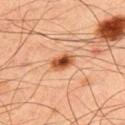Clinical impression: The lesion was photographed on a routine skin check and not biopsied; there is no pathology result. Context: The lesion is located on the upper back. The lesion-visualizer software estimated roughly 17 lightness units darker than nearby skin and a lesion-to-skin contrast of about 11 (normalized; higher = more distinct). The analysis additionally found border irregularity of about 1.5 on a 0–10 scale and a color-variation rating of about 5.5/10. The software also gave lesion-presence confidence of about 100/100. Imaged with cross-polarized lighting. A male patient, approximately 50 years of age. The lesion's longest dimension is about 3 mm. A roughly 15 mm field-of-view crop from a total-body skin photograph.